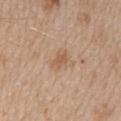lesion diameter: about 2.5 mm
patient: female, aged 43 to 47
TBP lesion metrics: a lesion area of about 3.5 mm², a shape eccentricity near 0.75, and two-axis asymmetry of about 0.3; about 8 CIELAB-L* units darker than the surrounding skin and a normalized border contrast of about 6; a nevus-likeness score of about 10/100
location: the upper back
lighting: white-light illumination
imaging modality: 15 mm crop, total-body photography